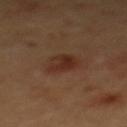image:
  source: total-body photography crop
  field_of_view_mm: 15
patient:
  sex: female
  age_approx: 40
lighting: cross-polarized
site: upper back
lesion_size:
  long_diameter_mm_approx: 3.5